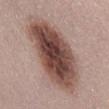{
  "biopsy_status": "not biopsied; imaged during a skin examination",
  "lesion_size": {
    "long_diameter_mm_approx": 11.0
  },
  "automated_metrics": {
    "area_mm2_approx": 45.0,
    "eccentricity": 0.85,
    "shape_asymmetry": 0.15,
    "color_variation_0_10": 9.0,
    "lesion_detection_confidence_0_100": 100
  },
  "site": "abdomen",
  "image": {
    "source": "total-body photography crop",
    "field_of_view_mm": 15
  },
  "lighting": "white-light",
  "patient": {
    "sex": "female",
    "age_approx": 50
  }
}